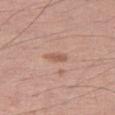notes — no biopsy performed (imaged during a skin exam)
patient — male, aged around 50
automated lesion analysis — an average lesion color of about L≈57 a*≈22 b*≈28 (CIELAB), a lesion–skin lightness drop of about 9, and a normalized lesion–skin contrast near 6.5; a classifier nevus-likeness of about 50/100 and lesion-presence confidence of about 100/100
lighting — white-light
image — 15 mm crop, total-body photography
diameter — about 2.5 mm
site — the right thigh